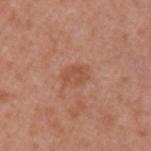Located on the left upper arm.
Imaged with white-light lighting.
A male patient, aged around 55.
The total-body-photography lesion software estimated an area of roughly 4 mm², an outline eccentricity of about 0.75 (0 = round, 1 = elongated), and two-axis asymmetry of about 0.25.
A 15 mm crop from a total-body photograph taken for skin-cancer surveillance.
Longest diameter approximately 3 mm.